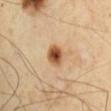The lesion was photographed on a routine skin check and not biopsied; there is no pathology result. A male subject aged around 65. The lesion is on the arm. A roughly 15 mm field-of-view crop from a total-body skin photograph.